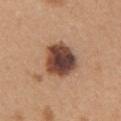Clinical impression: This lesion was catalogued during total-body skin photography and was not selected for biopsy. Image and clinical context: The subject is a female aged 43 to 47. From the left upper arm. The recorded lesion diameter is about 4.5 mm. Automated image analysis of the tile measured a lesion area of about 15 mm², a shape eccentricity near 0.55, and a shape-asymmetry score of about 0.2 (0 = symmetric). And it measured an average lesion color of about L≈44 a*≈20 b*≈27 (CIELAB), roughly 20 lightness units darker than nearby skin, and a normalized lesion–skin contrast near 14. It also reported border irregularity of about 2 on a 0–10 scale, a color-variation rating of about 7/10, and a peripheral color-asymmetry measure near 1.5. And it measured a classifier nevus-likeness of about 30/100 and lesion-presence confidence of about 100/100. Cropped from a whole-body photographic skin survey; the tile spans about 15 mm.